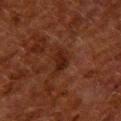Captured during whole-body skin photography for melanoma surveillance; the lesion was not biopsied. A female patient roughly 50 years of age. A 15 mm close-up extracted from a 3D total-body photography capture. The lesion is located on the upper back. This is a cross-polarized tile. The lesion's longest dimension is about 3.5 mm.